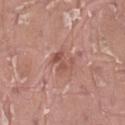Captured during whole-body skin photography for melanoma surveillance; the lesion was not biopsied.
The patient is a male about 40 years old.
Longest diameter approximately 3 mm.
Automated image analysis of the tile measured a shape eccentricity near 0.7 and a shape-asymmetry score of about 0.55 (0 = symmetric). The analysis additionally found a classifier nevus-likeness of about 0/100 and a detector confidence of about 100 out of 100 that the crop contains a lesion.
The lesion is on the left thigh.
A roughly 15 mm field-of-view crop from a total-body skin photograph.